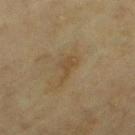The lesion was tiled from a total-body skin photograph and was not biopsied. The lesion's longest dimension is about 3.5 mm. Captured under cross-polarized illumination. A female subject, aged around 55. A 15 mm close-up tile from a total-body photography series done for melanoma screening. Located on the left thigh. An algorithmic analysis of the crop reported an outline eccentricity of about 0.9 (0 = round, 1 = elongated) and two-axis asymmetry of about 0.55. And it measured a normalized lesion–skin contrast near 5.5.